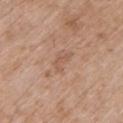Assessment: No biopsy was performed on this lesion — it was imaged during a full skin examination and was not determined to be concerning. Clinical summary: The patient is a female aged 73–77. This is a white-light tile. The lesion is located on the left upper arm. Cropped from a whole-body photographic skin survey; the tile spans about 15 mm.